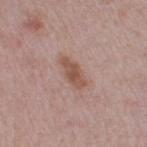notes: no biopsy performed (imaged during a skin exam); patient: female, about 30 years old; location: the right thigh; acquisition: ~15 mm tile from a whole-body skin photo.